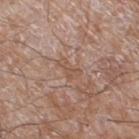Impression: The lesion was photographed on a routine skin check and not biopsied; there is no pathology result. Image and clinical context: Cropped from a total-body skin-imaging series; the visible field is about 15 mm. Imaged with white-light lighting. About 3 mm across. A male patient, about 60 years old. On the leg.